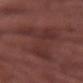follow-up=imaged on a skin check; not biopsied | TBP lesion metrics=a lesion area of about 31 mm², a shape eccentricity near 0.65, and a symmetry-axis asymmetry near 0.6; a lesion color around L≈33 a*≈22 b*≈20 in CIELAB and about 5 CIELAB-L* units darker than the surrounding skin; a within-lesion color-variation index near 3/10 and peripheral color asymmetry of about 1; an automated nevus-likeness rating near 0 out of 100 and lesion-presence confidence of about 85/100 | illumination=white-light illumination | body site=the right forearm | lesion diameter=≈9.5 mm | acquisition=15 mm crop, total-body photography | subject=male, aged 73 to 77.